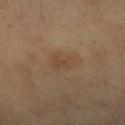Captured during whole-body skin photography for melanoma surveillance; the lesion was not biopsied.
An algorithmic analysis of the crop reported a shape eccentricity near 0.8 and a symmetry-axis asymmetry near 0.35. And it measured about 5 CIELAB-L* units darker than the surrounding skin. It also reported border irregularity of about 3.5 on a 0–10 scale, a color-variation rating of about 2/10, and peripheral color asymmetry of about 0.5. The software also gave an automated nevus-likeness rating near 0 out of 100.
Located on the arm.
A female subject, aged approximately 60.
The tile uses cross-polarized illumination.
The recorded lesion diameter is about 3.5 mm.
This image is a 15 mm lesion crop taken from a total-body photograph.